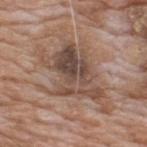Clinical impression:
Recorded during total-body skin imaging; not selected for excision or biopsy.
Clinical summary:
Cropped from a total-body skin-imaging series; the visible field is about 15 mm. Automated image analysis of the tile measured border irregularity of about 6 on a 0–10 scale, a color-variation rating of about 8.5/10, and a peripheral color-asymmetry measure near 3. Located on the upper back. This is a white-light tile. The patient is a male aged 58 to 62.